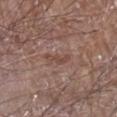Part of a total-body skin-imaging series; this lesion was reviewed on a skin check and was not flagged for biopsy. The lesion is on the arm. Automated tile analysis of the lesion measured a border-irregularity index near 7.5/10, a color-variation rating of about 0/10, and peripheral color asymmetry of about 0. The recorded lesion diameter is about 3 mm. A male patient aged 78–82. This is a white-light tile. A close-up tile cropped from a whole-body skin photograph, about 15 mm across.